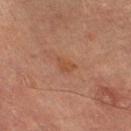The lesion was photographed on a routine skin check and not biopsied; there is no pathology result. This image is a 15 mm lesion crop taken from a total-body photograph. A male patient aged 83 or over. On the right lower leg. Imaged with cross-polarized lighting. Longest diameter approximately 2.5 mm.